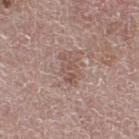Impression:
Part of a total-body skin-imaging series; this lesion was reviewed on a skin check and was not flagged for biopsy.
Background:
A close-up tile cropped from a whole-body skin photograph, about 15 mm across. From the right thigh. Captured under white-light illumination. The lesion-visualizer software estimated an area of roughly 5 mm², an outline eccentricity of about 0.85 (0 = round, 1 = elongated), and a symmetry-axis asymmetry near 0.3. It also reported a border-irregularity index near 4.5/10, a within-lesion color-variation index near 2.5/10, and radial color variation of about 1. The software also gave an automated nevus-likeness rating near 0 out of 100 and a lesion-detection confidence of about 100/100. Longest diameter approximately 4 mm. The patient is a female aged 63 to 67.